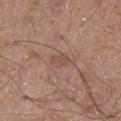Captured during whole-body skin photography for melanoma surveillance; the lesion was not biopsied. The lesion is located on the left thigh. Cropped from a whole-body photographic skin survey; the tile spans about 15 mm. Longest diameter approximately 2.5 mm. The tile uses white-light illumination. A male patient roughly 55 years of age.